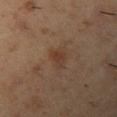The lesion was tiled from a total-body skin photograph and was not biopsied. The recorded lesion diameter is about 2.5 mm. A roughly 15 mm field-of-view crop from a total-body skin photograph. Located on the left upper arm. The subject is a male aged 53 to 57. Imaged with cross-polarized lighting.